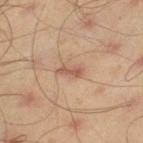Findings:
– image source — ~15 mm crop, total-body skin-cancer survey
– patient — male, aged 43 to 47
– TBP lesion metrics — a lesion area of about 3 mm², an eccentricity of roughly 0.9, and a symmetry-axis asymmetry near 0.3; an average lesion color of about L≈55 a*≈22 b*≈29 (CIELAB), about 10 CIELAB-L* units darker than the surrounding skin, and a normalized lesion–skin contrast near 7; border irregularity of about 3.5 on a 0–10 scale, a within-lesion color-variation index near 0/10, and a peripheral color-asymmetry measure near 0
– location — the left thigh
– illumination — cross-polarized illumination